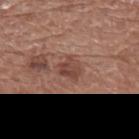Impression: Imaged during a routine full-body skin examination; the lesion was not biopsied and no histopathology is available. Image and clinical context: Captured under white-light illumination. A 15 mm crop from a total-body photograph taken for skin-cancer surveillance. Measured at roughly 2.5 mm in maximum diameter. The subject is a male about 75 years old. The lesion is located on the mid back. The lesion-visualizer software estimated an eccentricity of roughly 0.5. The analysis additionally found an average lesion color of about L≈44 a*≈22 b*≈25 (CIELAB) and a normalized border contrast of about 7. And it measured border irregularity of about 3.5 on a 0–10 scale and peripheral color asymmetry of about 1.5.